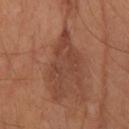No biopsy was performed on this lesion — it was imaged during a full skin examination and was not determined to be concerning. A female patient aged 58–62. A lesion tile, about 15 mm wide, cut from a 3D total-body photograph. The lesion is located on the right forearm.